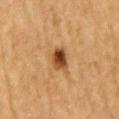Assessment:
No biopsy was performed on this lesion — it was imaged during a full skin examination and was not determined to be concerning.
Acquisition and patient details:
A male subject aged 83 or older. Located on the mid back. Automated image analysis of the tile measured a within-lesion color-variation index near 8.5/10 and a peripheral color-asymmetry measure near 3. A region of skin cropped from a whole-body photographic capture, roughly 15 mm wide. Longest diameter approximately 3 mm. The tile uses cross-polarized illumination.